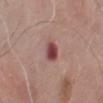Notes:
– follow-up: catalogued during a skin exam; not biopsied
– patient: male, aged around 80
– site: the chest
– acquisition: 15 mm crop, total-body photography